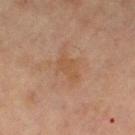Background: Cropped from a total-body skin-imaging series; the visible field is about 15 mm. A female patient aged 68–72. The lesion-visualizer software estimated an average lesion color of about L≈45 a*≈18 b*≈30 (CIELAB), a lesion–skin lightness drop of about 5, and a lesion-to-skin contrast of about 5 (normalized; higher = more distinct). It also reported a border-irregularity rating of about 2.5/10 and peripheral color asymmetry of about 0.5. The software also gave an automated nevus-likeness rating near 0 out of 100. The lesion is on the right thigh. Approximately 3 mm at its widest.